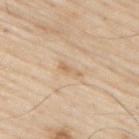Part of a total-body skin-imaging series; this lesion was reviewed on a skin check and was not flagged for biopsy. The patient is a male in their 80s. Captured under white-light illumination. A 15 mm close-up extracted from a 3D total-body photography capture. Located on the upper back. Automated image analysis of the tile measured a footprint of about 2.5 mm², a shape eccentricity near 0.95, and a shape-asymmetry score of about 0.4 (0 = symmetric).